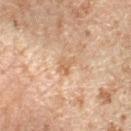This lesion was catalogued during total-body skin photography and was not selected for biopsy. From the right forearm. A male subject aged 43–47. Captured under cross-polarized illumination. Measured at roughly 2.5 mm in maximum diameter. This image is a 15 mm lesion crop taken from a total-body photograph. The total-body-photography lesion software estimated an outline eccentricity of about 0.75 (0 = round, 1 = elongated) and two-axis asymmetry of about 0.45. The analysis additionally found border irregularity of about 4.5 on a 0–10 scale, a color-variation rating of about 2/10, and radial color variation of about 0.5.